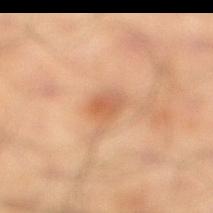biopsy status = catalogued during a skin exam; not biopsied
size = ~3 mm (longest diameter)
acquisition = total-body-photography crop, ~15 mm field of view
lighting = cross-polarized
body site = the right lower leg
patient = male, in their mid- to late 60s